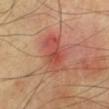Imaged during a routine full-body skin examination; the lesion was not biopsied and no histopathology is available.
The lesion is on the left lower leg.
A lesion tile, about 15 mm wide, cut from a 3D total-body photograph.
A male patient, aged approximately 70.